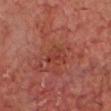This lesion was catalogued during total-body skin photography and was not selected for biopsy. About 2.5 mm across. A close-up tile cropped from a whole-body skin photograph, about 15 mm across. An algorithmic analysis of the crop reported a lesion area of about 4.5 mm², an outline eccentricity of about 0.65 (0 = round, 1 = elongated), and a symmetry-axis asymmetry near 0.4. It also reported an average lesion color of about L≈30 a*≈24 b*≈24 (CIELAB) and about 5 CIELAB-L* units darker than the surrounding skin. And it measured a border-irregularity index near 3.5/10 and a within-lesion color-variation index near 1.5/10. From the head or neck. A male subject roughly 65 years of age.